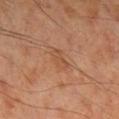The lesion is on the right lower leg.
A 15 mm crop from a total-body photograph taken for skin-cancer surveillance.
An algorithmic analysis of the crop reported a footprint of about 4.5 mm², an eccentricity of roughly 0.8, and a symmetry-axis asymmetry near 0.25. The analysis additionally found an average lesion color of about L≈49 a*≈22 b*≈33 (CIELAB) and roughly 6 lightness units darker than nearby skin. It also reported a border-irregularity index near 3/10, a within-lesion color-variation index near 2/10, and radial color variation of about 0.5. The software also gave an automated nevus-likeness rating near 0 out of 100 and lesion-presence confidence of about 100/100.
A male subject aged around 70.
Approximately 3 mm at its widest.
Imaged with cross-polarized lighting.